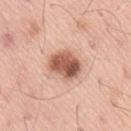Recorded during total-body skin imaging; not selected for excision or biopsy. The tile uses white-light illumination. The recorded lesion diameter is about 4.5 mm. Cropped from a whole-body photographic skin survey; the tile spans about 15 mm. A male patient in their 60s. The lesion is located on the lower back.